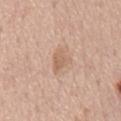biopsy status = imaged on a skin check; not biopsied | lighting = white-light illumination | diameter = ≈3.5 mm | image source = ~15 mm tile from a whole-body skin photo | patient = male, in their mid- to late 70s | body site = the chest.